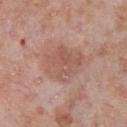workup: total-body-photography surveillance lesion; no biopsy
patient: male, aged approximately 80
lesion diameter: about 5 mm
image source: ~15 mm crop, total-body skin-cancer survey
site: the front of the torso
illumination: white-light
image-analysis metrics: two-axis asymmetry of about 0.1; a border-irregularity index near 1.5/10, internal color variation of about 3.5 on a 0–10 scale, and peripheral color asymmetry of about 1